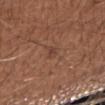Imaged during a routine full-body skin examination; the lesion was not biopsied and no histopathology is available.
Cropped from a total-body skin-imaging series; the visible field is about 15 mm.
Measured at roughly 2.5 mm in maximum diameter.
Automated tile analysis of the lesion measured an area of roughly 2.5 mm², an eccentricity of roughly 0.9, and two-axis asymmetry of about 0.25. The analysis additionally found a mean CIELAB color near L≈42 a*≈20 b*≈26, a lesion–skin lightness drop of about 6, and a normalized border contrast of about 5.5.
The lesion is on the right upper arm.
A male subject aged around 65.